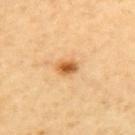| key | value |
|---|---|
| follow-up | no biopsy performed (imaged during a skin exam) |
| diameter | ~2.5 mm (longest diameter) |
| patient | female, about 60 years old |
| automated lesion analysis | a footprint of about 4 mm² and a shape eccentricity near 0.75 |
| tile lighting | cross-polarized |
| acquisition | ~15 mm tile from a whole-body skin photo |
| body site | the upper back |